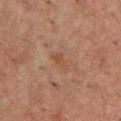Assessment:
Imaged during a routine full-body skin examination; the lesion was not biopsied and no histopathology is available.
Background:
Located on the chest. A region of skin cropped from a whole-body photographic capture, roughly 15 mm wide. Approximately 2.5 mm at its widest. Imaged with cross-polarized lighting. A male patient aged around 50.